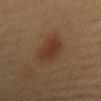workup: total-body-photography surveillance lesion; no biopsy | patient: female, in their mid- to late 40s | lighting: cross-polarized illumination | location: the back | acquisition: ~15 mm tile from a whole-body skin photo | lesion size: about 4.5 mm.